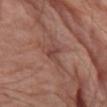workup = total-body-photography surveillance lesion; no biopsy | tile lighting = white-light illumination | image = ~15 mm crop, total-body skin-cancer survey | patient = male, in their 80s | body site = the right upper arm | image-analysis metrics = a shape eccentricity near 0.9; a within-lesion color-variation index near 1/10 and a peripheral color-asymmetry measure near 0; a nevus-likeness score of about 0/100 and a detector confidence of about 55 out of 100 that the crop contains a lesion.